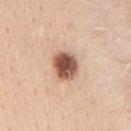Imaged during a routine full-body skin examination; the lesion was not biopsied and no histopathology is available.
Longest diameter approximately 3.5 mm.
The subject is a male aged around 30.
Located on the mid back.
Cropped from a total-body skin-imaging series; the visible field is about 15 mm.